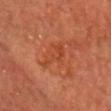Part of a total-body skin-imaging series; this lesion was reviewed on a skin check and was not flagged for biopsy. The lesion is on the head or neck. This image is a 15 mm lesion crop taken from a total-body photograph. A male patient aged approximately 65.